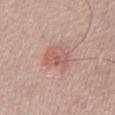This lesion was catalogued during total-body skin photography and was not selected for biopsy. A 15 mm close-up tile from a total-body photography series done for melanoma screening. A male patient, aged 58–62. Approximately 4 mm at its widest. The lesion is on the left lower leg. This is a white-light tile.